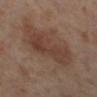Findings:
* follow-up · imaged on a skin check; not biopsied
* TBP lesion metrics · a lesion color around L≈39 a*≈18 b*≈25 in CIELAB and a normalized lesion–skin contrast near 6; a border-irregularity rating of about 4/10, a color-variation rating of about 3.5/10, and peripheral color asymmetry of about 1
* size · ≈6 mm
* image source · total-body-photography crop, ~15 mm field of view
* location · the left lower leg
* lighting · cross-polarized
* patient · female, aged 53–57